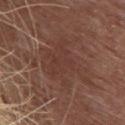follow-up=catalogued during a skin exam; not biopsied
subject=female, roughly 80 years of age
size=~7 mm (longest diameter)
anatomic site=the upper back
TBP lesion metrics=a lesion area of about 21 mm², an eccentricity of roughly 0.75, and two-axis asymmetry of about 0.4; about 5 CIELAB-L* units darker than the surrounding skin and a normalized lesion–skin contrast near 4.5; an automated nevus-likeness rating near 0 out of 100 and a detector confidence of about 80 out of 100 that the crop contains a lesion
imaging modality=total-body-photography crop, ~15 mm field of view